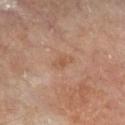follow-up = catalogued during a skin exam; not biopsied | automated metrics = a footprint of about 2.5 mm², a shape eccentricity near 0.85, and two-axis asymmetry of about 0.45; an automated nevus-likeness rating near 0 out of 100 and a detector confidence of about 100 out of 100 that the crop contains a lesion | acquisition = total-body-photography crop, ~15 mm field of view | location = the right lower leg | patient = male, aged 63 to 67 | diameter = ~2.5 mm (longest diameter).